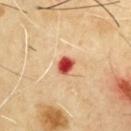A 15 mm close-up extracted from a 3D total-body photography capture.
The total-body-photography lesion software estimated an area of roughly 4 mm², a shape eccentricity near 0.6, and a shape-asymmetry score of about 0.15 (0 = symmetric). And it measured a lesion-to-skin contrast of about 13 (normalized; higher = more distinct). And it measured a border-irregularity rating of about 1.5/10 and internal color variation of about 3 on a 0–10 scale.
The recorded lesion diameter is about 2.5 mm.
From the chest.
A male patient aged 63–67.
Imaged with cross-polarized lighting.